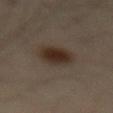<tbp_lesion>
  <biopsy_status>not biopsied; imaged during a skin examination</biopsy_status>
  <patient>
    <sex>male</sex>
    <age_approx>55</age_approx>
  </patient>
  <lighting>cross-polarized</lighting>
  <image>
    <source>total-body photography crop</source>
    <field_of_view_mm>15</field_of_view_mm>
  </image>
  <site>leg</site>
</tbp_lesion>